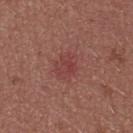Part of a total-body skin-imaging series; this lesion was reviewed on a skin check and was not flagged for biopsy.
A female subject aged 23–27.
Captured under white-light illumination.
From the upper back.
This image is a 15 mm lesion crop taken from a total-body photograph.
The lesion's longest dimension is about 4.5 mm.